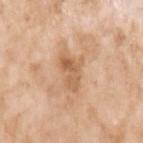Assessment:
The lesion was photographed on a routine skin check and not biopsied; there is no pathology result.
Context:
The lesion's longest dimension is about 4 mm. The subject is a female in their mid- to late 70s. The lesion is on the left upper arm. Imaged with white-light lighting. A lesion tile, about 15 mm wide, cut from a 3D total-body photograph. Automated image analysis of the tile measured a lesion area of about 7 mm², an eccentricity of roughly 0.8, and two-axis asymmetry of about 0.45.